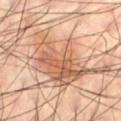* workup · catalogued during a skin exam; not biopsied
* site · the left thigh
* lighting · cross-polarized illumination
* TBP lesion metrics · peripheral color asymmetry of about 2.5
* acquisition · ~15 mm tile from a whole-body skin photo
* patient · male, aged 58 to 62